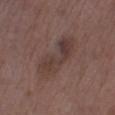Clinical summary:
A male subject roughly 75 years of age. An algorithmic analysis of the crop reported a shape eccentricity near 0.95 and a shape-asymmetry score of about 0.45 (0 = symmetric). The analysis additionally found a mean CIELAB color near L≈37 a*≈16 b*≈20 and a lesion–skin lightness drop of about 7. It also reported a nevus-likeness score of about 0/100 and lesion-presence confidence of about 100/100. The lesion is on the leg. This image is a 15 mm lesion crop taken from a total-body photograph. Captured under white-light illumination.